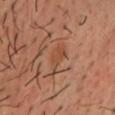Captured during whole-body skin photography for melanoma surveillance; the lesion was not biopsied.
The total-body-photography lesion software estimated a border-irregularity rating of about 4.5/10, a within-lesion color-variation index near 2.5/10, and a peripheral color-asymmetry measure near 1. The analysis additionally found an automated nevus-likeness rating near 5 out of 100 and lesion-presence confidence of about 100/100.
The lesion's longest dimension is about 4.5 mm.
The patient is a male aged 38 to 42.
A 15 mm crop from a total-body photograph taken for skin-cancer surveillance.
The tile uses cross-polarized illumination.
Located on the chest.